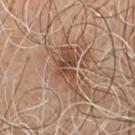Clinical impression:
Part of a total-body skin-imaging series; this lesion was reviewed on a skin check and was not flagged for biopsy.
Context:
From the chest. A roughly 15 mm field-of-view crop from a total-body skin photograph. This is a cross-polarized tile. A male patient about 55 years old. Automated image analysis of the tile measured an average lesion color of about L≈47 a*≈19 b*≈29 (CIELAB) and a normalized border contrast of about 8. The software also gave a border-irregularity rating of about 6/10, a color-variation rating of about 5/10, and a peripheral color-asymmetry measure near 1.5. The analysis additionally found a lesion-detection confidence of about 100/100. The lesion's longest dimension is about 5.5 mm.